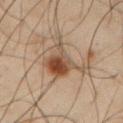No biopsy was performed on this lesion — it was imaged during a full skin examination and was not determined to be concerning. The tile uses cross-polarized illumination. The recorded lesion diameter is about 8 mm. A male subject roughly 50 years of age. Automated image analysis of the tile measured a lesion area of about 16 mm², an eccentricity of roughly 0.9, and a symmetry-axis asymmetry near 0.55. The analysis additionally found border irregularity of about 7.5 on a 0–10 scale, a color-variation rating of about 8.5/10, and radial color variation of about 2.5. It also reported a lesion-detection confidence of about 100/100. Located on the right upper arm. This image is a 15 mm lesion crop taken from a total-body photograph.